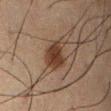biopsy status — catalogued during a skin exam; not biopsied | image — ~15 mm tile from a whole-body skin photo | subject — male, approximately 60 years of age | diameter — ≈3.5 mm | body site — the abdomen | lighting — cross-polarized.